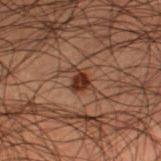| key | value |
|---|---|
| anatomic site | the left lower leg |
| patient | male, aged 48 to 52 |
| imaging modality | ~15 mm crop, total-body skin-cancer survey |